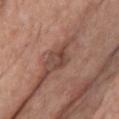This lesion was catalogued during total-body skin photography and was not selected for biopsy.
The lesion is on the chest.
Captured under white-light illumination.
Approximately 3 mm at its widest.
The patient is a female roughly 85 years of age or older.
Cropped from a total-body skin-imaging series; the visible field is about 15 mm.